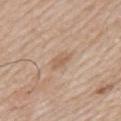Captured during whole-body skin photography for melanoma surveillance; the lesion was not biopsied. Longest diameter approximately 2.5 mm. Automated tile analysis of the lesion measured a lesion area of about 4 mm², a shape eccentricity near 0.75, and a symmetry-axis asymmetry near 0.25. The software also gave a border-irregularity rating of about 2.5/10, a color-variation rating of about 1.5/10, and a peripheral color-asymmetry measure near 0.5. The lesion is located on the mid back. This is a white-light tile. A male subject, roughly 60 years of age. A 15 mm close-up extracted from a 3D total-body photography capture.